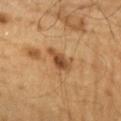Notes:
– workup — catalogued during a skin exam; not biopsied
– patient — male, in their 60s
– size — ~3.5 mm (longest diameter)
– image — total-body-photography crop, ~15 mm field of view
– anatomic site — the chest
– illumination — cross-polarized illumination